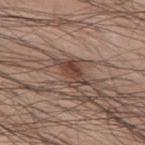{"automated_metrics": {"nevus_likeness_0_100": 90, "lesion_detection_confidence_0_100": 100}, "image": {"source": "total-body photography crop", "field_of_view_mm": 15}, "lesion_size": {"long_diameter_mm_approx": 3.5}, "site": "upper back", "patient": {"sex": "male", "age_approx": 60}}